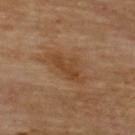follow-up — catalogued during a skin exam; not biopsied | image — ~15 mm tile from a whole-body skin photo | subject — male, aged 68 to 72 | lesion diameter — ≈5 mm | anatomic site — the upper back | image-analysis metrics — an area of roughly 7 mm², an outline eccentricity of about 0.9 (0 = round, 1 = elongated), and a shape-asymmetry score of about 0.35 (0 = symmetric); an average lesion color of about L≈42 a*≈20 b*≈33 (CIELAB) and a lesion–skin lightness drop of about 7; border irregularity of about 4.5 on a 0–10 scale, a color-variation rating of about 2.5/10, and radial color variation of about 0.5; an automated nevus-likeness rating near 0 out of 100.